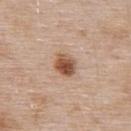Captured during whole-body skin photography for melanoma surveillance; the lesion was not biopsied.
The recorded lesion diameter is about 3 mm.
The lesion-visualizer software estimated a border-irregularity index near 1.5/10, internal color variation of about 5.5 on a 0–10 scale, and a peripheral color-asymmetry measure near 1.5. And it measured a lesion-detection confidence of about 100/100.
From the back.
The tile uses white-light illumination.
A region of skin cropped from a whole-body photographic capture, roughly 15 mm wide.
A male patient, in their mid- to late 50s.